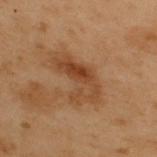Assessment: The lesion was tiled from a total-body skin photograph and was not biopsied. Image and clinical context: Cropped from a whole-body photographic skin survey; the tile spans about 15 mm. The lesion is located on the upper back. About 6 mm across. The subject is a male approximately 55 years of age. The tile uses cross-polarized illumination.